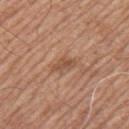Captured during whole-body skin photography for melanoma surveillance; the lesion was not biopsied.
The recorded lesion diameter is about 2.5 mm.
The tile uses white-light illumination.
This image is a 15 mm lesion crop taken from a total-body photograph.
The lesion-visualizer software estimated a footprint of about 3.5 mm², a shape eccentricity near 0.85, and a shape-asymmetry score of about 0.4 (0 = symmetric). The software also gave an automated nevus-likeness rating near 0 out of 100.
The subject is a male aged 63 to 67.
The lesion is located on the arm.